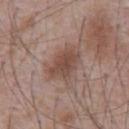Imaged during a routine full-body skin examination; the lesion was not biopsied and no histopathology is available. A 15 mm close-up tile from a total-body photography series done for melanoma screening. Imaged with white-light lighting. Automated image analysis of the tile measured a footprint of about 13 mm², a shape eccentricity near 0.75, and a symmetry-axis asymmetry near 0.25. It also reported a lesion color around L≈48 a*≈18 b*≈24 in CIELAB and a lesion–skin lightness drop of about 9. And it measured a detector confidence of about 100 out of 100 that the crop contains a lesion. A male subject aged around 70. Measured at roughly 5 mm in maximum diameter. On the abdomen.